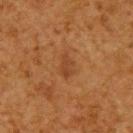| key | value |
|---|---|
| follow-up | no biopsy performed (imaged during a skin exam) |
| site | the upper back |
| imaging modality | ~15 mm tile from a whole-body skin photo |
| patient | male, in their 60s |